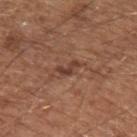biopsy status: total-body-photography surveillance lesion; no biopsy
subject: male, about 65 years old
TBP lesion metrics: a classifier nevus-likeness of about 0/100 and lesion-presence confidence of about 85/100
image: ~15 mm crop, total-body skin-cancer survey
lesion size: about 3 mm
tile lighting: white-light illumination
anatomic site: the left upper arm